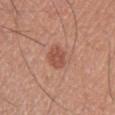  biopsy_status: not biopsied; imaged during a skin examination
  patient:
    sex: male
    age_approx: 40
  lesion_size:
    long_diameter_mm_approx: 3.0
  image:
    source: total-body photography crop
    field_of_view_mm: 15
  site: chest
  lighting: white-light